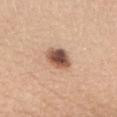workup=imaged on a skin check; not biopsied | automated metrics=an area of roughly 7 mm², a shape eccentricity near 0.65, and a symmetry-axis asymmetry near 0.15; roughly 19 lightness units darker than nearby skin and a normalized lesion–skin contrast near 12; a border-irregularity index near 1.5/10, a within-lesion color-variation index near 6.5/10, and radial color variation of about 1.5; an automated nevus-likeness rating near 95 out of 100 and a detector confidence of about 100 out of 100 that the crop contains a lesion | lighting=white-light | imaging modality=15 mm crop, total-body photography | subject=female, aged around 65 | body site=the lower back.